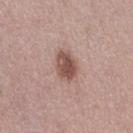workup = catalogued during a skin exam; not biopsied
subject = female, aged 38–42
location = the right thigh
image source = 15 mm crop, total-body photography
diameter = ~3.5 mm (longest diameter)